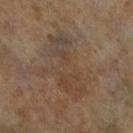size = ≈8.5 mm | lighting = cross-polarized illumination | automated metrics = a lesion area of about 20 mm² and a shape eccentricity near 0.95; an average lesion color of about L≈40 a*≈13 b*≈25 (CIELAB) and about 6 CIELAB-L* units darker than the surrounding skin; border irregularity of about 6.5 on a 0–10 scale, a within-lesion color-variation index near 6/10, and radial color variation of about 2; an automated nevus-likeness rating near 0 out of 100 and a detector confidence of about 80 out of 100 that the crop contains a lesion | imaging modality = ~15 mm crop, total-body skin-cancer survey | location = the left leg | patient = female, in their mid- to late 60s.